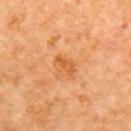workup: no biopsy performed (imaged during a skin exam); tile lighting: cross-polarized; site: the upper back; image source: ~15 mm crop, total-body skin-cancer survey; lesion size: ~3 mm (longest diameter); patient: female, aged 48 to 52.